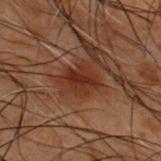Clinical impression: The lesion was tiled from a total-body skin photograph and was not biopsied. Clinical summary: The subject is a male aged 48–52. On the upper back. This image is a 15 mm lesion crop taken from a total-body photograph.